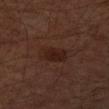From the left forearm.
The lesion-visualizer software estimated an area of roughly 7.5 mm² and an eccentricity of roughly 0.8. And it measured an average lesion color of about L≈19 a*≈17 b*≈20 (CIELAB) and a normalized border contrast of about 7. The software also gave an automated nevus-likeness rating near 30 out of 100 and a detector confidence of about 100 out of 100 that the crop contains a lesion.
Longest diameter approximately 4 mm.
This image is a 15 mm lesion crop taken from a total-body photograph.
A male patient aged approximately 60.
Captured under cross-polarized illumination.